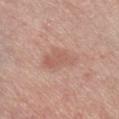The lesion was tiled from a total-body skin photograph and was not biopsied. Measured at roughly 5 mm in maximum diameter. This image is a 15 mm lesion crop taken from a total-body photograph. The subject is a male roughly 70 years of age. The total-body-photography lesion software estimated an area of roughly 10 mm² and a shape eccentricity near 0.85. On the right lower leg.